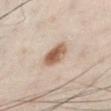The lesion was photographed on a routine skin check and not biopsied; there is no pathology result.
Located on the left lower leg.
This image is a 15 mm lesion crop taken from a total-body photograph.
About 3.5 mm across.
The tile uses cross-polarized illumination.
A male subject, roughly 50 years of age.